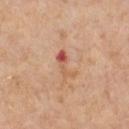Imaged during a routine full-body skin examination; the lesion was not biopsied and no histopathology is available. The lesion is on the chest. This is a white-light tile. A 15 mm close-up tile from a total-body photography series done for melanoma screening. The subject is a male approximately 60 years of age.